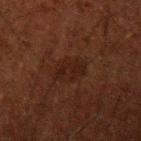Imaged during a routine full-body skin examination; the lesion was not biopsied and no histopathology is available.
The patient is a male aged 48 to 52.
This is a cross-polarized tile.
From the left upper arm.
About 4 mm across.
Cropped from a whole-body photographic skin survey; the tile spans about 15 mm.